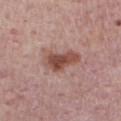Impression: The lesion was photographed on a routine skin check and not biopsied; there is no pathology result. Image and clinical context: A male subject, in their mid- to late 70s. The lesion is on the chest. Longest diameter approximately 4.5 mm. The total-body-photography lesion software estimated a nevus-likeness score of about 90/100 and a detector confidence of about 100 out of 100 that the crop contains a lesion. A lesion tile, about 15 mm wide, cut from a 3D total-body photograph.